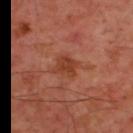- follow-up: no biopsy performed (imaged during a skin exam)
- illumination: cross-polarized illumination
- site: the upper back
- image source: total-body-photography crop, ~15 mm field of view
- subject: male, roughly 45 years of age